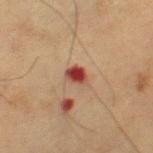workup=no biopsy performed (imaged during a skin exam)
subject=male, aged around 55
lesion diameter=~2.5 mm (longest diameter)
automated lesion analysis=a mean CIELAB color near L≈41 a*≈27 b*≈27, about 14 CIELAB-L* units darker than the surrounding skin, and a normalized lesion–skin contrast near 11; a nevus-likeness score of about 0/100
acquisition=~15 mm crop, total-body skin-cancer survey
anatomic site=the right thigh